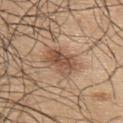notes: catalogued during a skin exam; not biopsied | anatomic site: the upper back | subject: male, roughly 45 years of age | illumination: white-light | image source: total-body-photography crop, ~15 mm field of view.